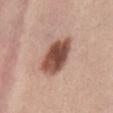<case>
  <image>
    <source>total-body photography crop</source>
    <field_of_view_mm>15</field_of_view_mm>
  </image>
  <lighting>white-light</lighting>
  <lesion_size>
    <long_diameter_mm_approx>5.5</long_diameter_mm_approx>
  </lesion_size>
  <patient>
    <sex>female</sex>
    <age_approx>35</age_approx>
  </patient>
  <site>abdomen</site>
</case>